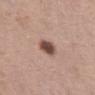<lesion>
<biopsy_status>not biopsied; imaged during a skin examination</biopsy_status>
<lighting>white-light</lighting>
<automated_metrics>
  <nevus_likeness_0_100>95</nevus_likeness_0_100>
  <lesion_detection_confidence_0_100>100</lesion_detection_confidence_0_100>
</automated_metrics>
<site>abdomen</site>
<patient>
  <sex>female</sex>
  <age_approx>40</age_approx>
</patient>
<image>
  <source>total-body photography crop</source>
  <field_of_view_mm>15</field_of_view_mm>
</image>
</lesion>